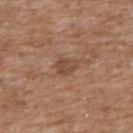Captured during whole-body skin photography for melanoma surveillance; the lesion was not biopsied. Located on the chest. This is a white-light tile. The total-body-photography lesion software estimated an automated nevus-likeness rating near 0 out of 100 and a lesion-detection confidence of about 100/100. About 2.5 mm across. A roughly 15 mm field-of-view crop from a total-body skin photograph. The patient is a male aged approximately 60.